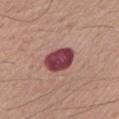notes = catalogued during a skin exam; not biopsied
lighting = white-light
site = the arm
subject = male, aged 58 to 62
imaging modality = ~15 mm tile from a whole-body skin photo
lesion diameter = about 3.5 mm